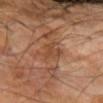The subject is a male aged approximately 70. A 15 mm crop from a total-body photograph taken for skin-cancer surveillance. About 4 mm across. Located on the left forearm.